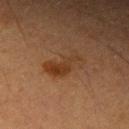Q: Is there a histopathology result?
A: no biopsy performed (imaged during a skin exam)
Q: What is the lesion's diameter?
A: ~5 mm (longest diameter)
Q: Illumination type?
A: cross-polarized illumination
Q: What kind of image is this?
A: ~15 mm crop, total-body skin-cancer survey
Q: Where on the body is the lesion?
A: the left upper arm
Q: What are the patient's age and sex?
A: male, aged 53–57